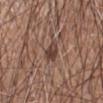| field | value |
|---|---|
| notes | imaged on a skin check; not biopsied |
| image source | 15 mm crop, total-body photography |
| body site | the left forearm |
| subject | male, aged approximately 60 |